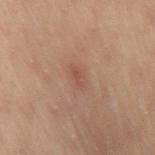Q: Was a biopsy performed?
A: imaged on a skin check; not biopsied
Q: Who is the patient?
A: female, aged 53–57
Q: Illumination type?
A: cross-polarized
Q: What is the anatomic site?
A: the lower back
Q: What is the imaging modality?
A: 15 mm crop, total-body photography
Q: What is the lesion's diameter?
A: ≈2.5 mm
Q: Automated lesion metrics?
A: a lesion color around L≈42 a*≈18 b*≈23 in CIELAB, roughly 5 lightness units darker than nearby skin, and a lesion-to-skin contrast of about 4.5 (normalized; higher = more distinct); a border-irregularity index near 2.5/10, internal color variation of about 2 on a 0–10 scale, and peripheral color asymmetry of about 1; a nevus-likeness score of about 0/100 and a detector confidence of about 100 out of 100 that the crop contains a lesion